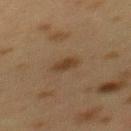follow-up: no biopsy performed (imaged during a skin exam) | illumination: cross-polarized illumination | acquisition: ~15 mm crop, total-body skin-cancer survey | body site: the mid back | patient: female, in their 40s | lesion size: about 3 mm | TBP lesion metrics: a shape eccentricity near 0.8 and a symmetry-axis asymmetry near 0.2.